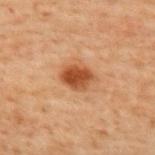follow-up: no biopsy performed (imaged during a skin exam)
automated lesion analysis: an area of roughly 7 mm², an outline eccentricity of about 0.6 (0 = round, 1 = elongated), and a symmetry-axis asymmetry near 0.15; border irregularity of about 1.5 on a 0–10 scale, internal color variation of about 3.5 on a 0–10 scale, and peripheral color asymmetry of about 1; a nevus-likeness score of about 100/100 and a detector confidence of about 100 out of 100 that the crop contains a lesion
tile lighting: cross-polarized illumination
diameter: about 3.5 mm
subject: male, approximately 70 years of age
body site: the mid back
image: 15 mm crop, total-body photography